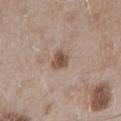Image and clinical context: An algorithmic analysis of the crop reported roughly 12 lightness units darker than nearby skin and a lesion-to-skin contrast of about 9 (normalized; higher = more distinct). The analysis additionally found border irregularity of about 3 on a 0–10 scale and a within-lesion color-variation index near 2.5/10. It also reported a nevus-likeness score of about 55/100 and lesion-presence confidence of about 100/100. The recorded lesion diameter is about 3 mm. Cropped from a total-body skin-imaging series; the visible field is about 15 mm. From the right upper arm. A male subject, about 55 years old. The tile uses white-light illumination.